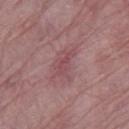Impression: The lesion was tiled from a total-body skin photograph and was not biopsied. Image and clinical context: This is a white-light tile. The recorded lesion diameter is about 3.5 mm. A 15 mm close-up extracted from a 3D total-body photography capture. From the right thigh. A female subject in their mid-60s.